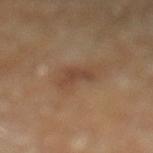Impression:
The lesion was photographed on a routine skin check and not biopsied; there is no pathology result.
Background:
The lesion is located on the left lower leg. The tile uses cross-polarized illumination. A male subject, aged 83 or over. A lesion tile, about 15 mm wide, cut from a 3D total-body photograph. About 3 mm across.